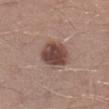Recorded during total-body skin imaging; not selected for excision or biopsy. The patient is a male aged approximately 30. The lesion is on the right lower leg. A 15 mm crop from a total-body photograph taken for skin-cancer surveillance.